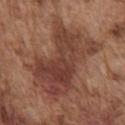The lesion was photographed on a routine skin check and not biopsied; there is no pathology result.
A male subject in their mid-70s.
From the front of the torso.
The lesion-visualizer software estimated a border-irregularity rating of about 5.5/10. The analysis additionally found a nevus-likeness score of about 5/100 and lesion-presence confidence of about 100/100.
A 15 mm close-up tile from a total-body photography series done for melanoma screening.
About 9.5 mm across.